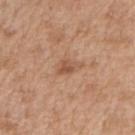Part of a total-body skin-imaging series; this lesion was reviewed on a skin check and was not flagged for biopsy. The lesion is located on the right upper arm. Automated image analysis of the tile measured a footprint of about 3.5 mm², a shape eccentricity near 0.75, and a symmetry-axis asymmetry near 0.35. The software also gave a mean CIELAB color near L≈54 a*≈21 b*≈32, roughly 9 lightness units darker than nearby skin, and a lesion-to-skin contrast of about 6.5 (normalized; higher = more distinct). Cropped from a whole-body photographic skin survey; the tile spans about 15 mm. The patient is a male roughly 70 years of age. The tile uses white-light illumination. Approximately 3 mm at its widest.